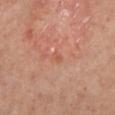biopsy status: no biopsy performed (imaged during a skin exam); lesion diameter: about 1.5 mm; automated metrics: a classifier nevus-likeness of about 0/100 and a lesion-detection confidence of about 100/100; anatomic site: the left lower leg; acquisition: 15 mm crop, total-body photography; illumination: cross-polarized; subject: male, aged 48–52.